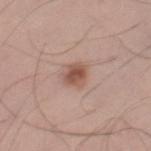notes — imaged on a skin check; not biopsied
image source — 15 mm crop, total-body photography
site — the left thigh
tile lighting — white-light illumination
subject — male, in their 40s
lesion size — ~3 mm (longest diameter)